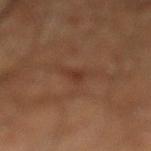Impression:
Captured during whole-body skin photography for melanoma surveillance; the lesion was not biopsied.
Background:
A male subject aged 58 to 62. Approximately 2 mm at its widest. The lesion is on the left lower leg. The tile uses cross-polarized illumination. A lesion tile, about 15 mm wide, cut from a 3D total-body photograph.